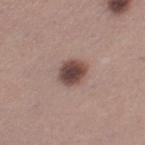Imaged during a routine full-body skin examination; the lesion was not biopsied and no histopathology is available. This is a white-light tile. On the left thigh. About 3 mm across. A female patient approximately 30 years of age. Cropped from a whole-body photographic skin survey; the tile spans about 15 mm.